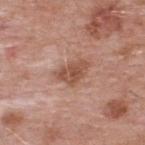follow-up: total-body-photography surveillance lesion; no biopsy | site: the upper back | image: ~15 mm tile from a whole-body skin photo | automated metrics: a classifier nevus-likeness of about 30/100 | lesion diameter: about 4 mm | patient: male, aged approximately 55 | lighting: white-light.